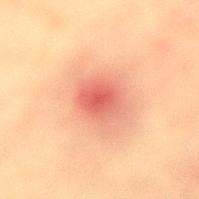Assessment:
Recorded during total-body skin imaging; not selected for excision or biopsy.
Acquisition and patient details:
Captured under cross-polarized illumination. A close-up tile cropped from a whole-body skin photograph, about 15 mm across. A female subject, about 40 years old. The lesion is located on the lower back. Approximately 3.5 mm at its widest.